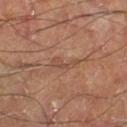  site: leg
  patient:
    sex: male
    age_approx: 70
  image:
    source: total-body photography crop
    field_of_view_mm: 15
  lighting: cross-polarized
  automated_metrics:
    vs_skin_darker_L: 7.0
    vs_skin_contrast_norm: 5.0
    color_variation_0_10: 0.0
    peripheral_color_asymmetry: 0.0